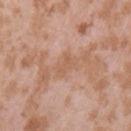Case summary:
* notes — catalogued during a skin exam; not biopsied
* patient — female, about 25 years old
* imaging modality — total-body-photography crop, ~15 mm field of view
* anatomic site — the left upper arm